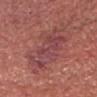This lesion was catalogued during total-body skin photography and was not selected for biopsy.
Longest diameter approximately 7.5 mm.
Automated tile analysis of the lesion measured border irregularity of about 8 on a 0–10 scale, internal color variation of about 5.5 on a 0–10 scale, and peripheral color asymmetry of about 2. And it measured a classifier nevus-likeness of about 0/100 and a detector confidence of about 95 out of 100 that the crop contains a lesion.
A roughly 15 mm field-of-view crop from a total-body skin photograph.
Located on the head or neck.
A male patient about 65 years old.